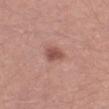{
  "biopsy_status": "not biopsied; imaged during a skin examination",
  "lesion_size": {
    "long_diameter_mm_approx": 2.5
  },
  "image": {
    "source": "total-body photography crop",
    "field_of_view_mm": 15
  },
  "patient": {
    "sex": "male",
    "age_approx": 45
  },
  "lighting": "white-light",
  "site": "left thigh"
}